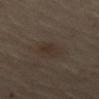{
  "biopsy_status": "not biopsied; imaged during a skin examination",
  "lesion_size": {
    "long_diameter_mm_approx": 3.0
  },
  "lighting": "cross-polarized",
  "image": {
    "source": "total-body photography crop",
    "field_of_view_mm": 15
  },
  "site": "front of the torso"
}